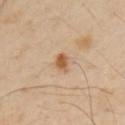Q: Was this lesion biopsied?
A: imaged on a skin check; not biopsied